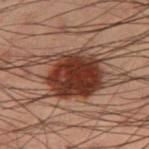Notes:
* body site — the right thigh
* image source — 15 mm crop, total-body photography
* patient — male, approximately 55 years of age